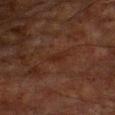follow-up: no biopsy performed (imaged during a skin exam); site: the chest; imaging modality: total-body-photography crop, ~15 mm field of view; size: about 3 mm; tile lighting: cross-polarized illumination; patient: male, approximately 65 years of age.